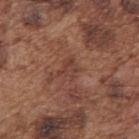A lesion tile, about 15 mm wide, cut from a 3D total-body photograph. From the right upper arm. This is a white-light tile. A male subject, aged approximately 75. Longest diameter approximately 2.5 mm.